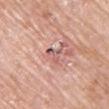The lesion is on the front of the torso.
A female subject, aged around 65.
Automated image analysis of the tile measured a lesion area of about 2.5 mm², an eccentricity of roughly 0.75, and a symmetry-axis asymmetry near 0.7. It also reported an average lesion color of about L≈57 a*≈23 b*≈23 (CIELAB), a lesion–skin lightness drop of about 11, and a lesion-to-skin contrast of about 7 (normalized; higher = more distinct). The software also gave a within-lesion color-variation index near 0/10. The analysis additionally found a lesion-detection confidence of about 100/100.
A 15 mm crop from a total-body photograph taken for skin-cancer surveillance.
Imaged with white-light lighting.
About 2.5 mm across.